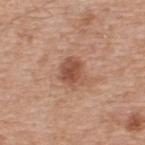Findings:
• follow-up: catalogued during a skin exam; not biopsied
• lesion diameter: ≈3.5 mm
• tile lighting: white-light illumination
• anatomic site: the upper back
• subject: male, about 55 years old
• image: 15 mm crop, total-body photography
• automated lesion analysis: an area of roughly 7 mm², a shape eccentricity near 0.5, and a shape-asymmetry score of about 0.2 (0 = symmetric); a nevus-likeness score of about 70/100 and lesion-presence confidence of about 100/100